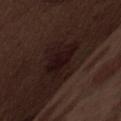Imaged during a routine full-body skin examination; the lesion was not biopsied and no histopathology is available. From the abdomen. A 15 mm close-up tile from a total-body photography series done for melanoma screening. The patient is a male aged around 70. The tile uses white-light illumination. Longest diameter approximately 5 mm. An algorithmic analysis of the crop reported a classifier nevus-likeness of about 5/100 and a detector confidence of about 60 out of 100 that the crop contains a lesion.